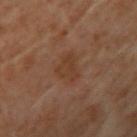biopsy status: imaged on a skin check; not biopsied | patient: female, aged around 60 | site: the upper back | image: ~15 mm crop, total-body skin-cancer survey | image-analysis metrics: a lesion area of about 7 mm², a shape eccentricity near 0.6, and a shape-asymmetry score of about 0.4 (0 = symmetric); a lesion color around L≈32 a*≈17 b*≈26 in CIELAB, a lesion–skin lightness drop of about 5, and a normalized lesion–skin contrast near 6; a border-irregularity index near 4/10 and a color-variation rating of about 2/10; a nevus-likeness score of about 0/100 and a lesion-detection confidence of about 100/100 | tile lighting: cross-polarized | size: ~3.5 mm (longest diameter).